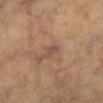{"biopsy_status": "not biopsied; imaged during a skin examination", "lighting": "cross-polarized", "patient": {"sex": "female", "age_approx": 60}, "lesion_size": {"long_diameter_mm_approx": 3.0}, "site": "left lower leg", "automated_metrics": {"cielab_L": 45, "cielab_a": 17, "cielab_b": 23, "vs_skin_darker_L": 6.0, "vs_skin_contrast_norm": 6.0, "border_irregularity_0_10": 3.0, "color_variation_0_10": 1.5, "peripheral_color_asymmetry": 0.5}, "image": {"source": "total-body photography crop", "field_of_view_mm": 15}}